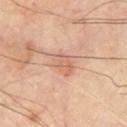| field | value |
|---|---|
| notes | total-body-photography surveillance lesion; no biopsy |
| automated metrics | an average lesion color of about L≈64 a*≈23 b*≈31 (CIELAB), about 8 CIELAB-L* units darker than the surrounding skin, and a normalized lesion–skin contrast near 5.5; a within-lesion color-variation index near 3/10 and a peripheral color-asymmetry measure near 1; a nevus-likeness score of about 15/100 |
| image | total-body-photography crop, ~15 mm field of view |
| location | the chest |
| lesion size | ≈3 mm |
| patient | male, aged 63–67 |